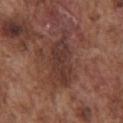notes: no biopsy performed (imaged during a skin exam) | image: 15 mm crop, total-body photography | automated lesion analysis: a lesion area of about 16 mm² and two-axis asymmetry of about 0.3; a lesion color around L≈36 a*≈20 b*≈23 in CIELAB, about 8 CIELAB-L* units darker than the surrounding skin, and a normalized border contrast of about 7.5; border irregularity of about 4 on a 0–10 scale, a color-variation rating of about 3.5/10, and radial color variation of about 1; an automated nevus-likeness rating near 0 out of 100 | diameter: ≈7 mm | site: the front of the torso | patient: male, aged 73 to 77.